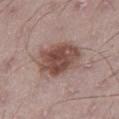Q: How was this image acquired?
A: ~15 mm crop, total-body skin-cancer survey
Q: Patient demographics?
A: male, aged approximately 65
Q: Where on the body is the lesion?
A: the left thigh